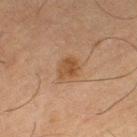biopsy_status: not biopsied; imaged during a skin examination
image:
  source: total-body photography crop
  field_of_view_mm: 15
patient:
  sex: male
  age_approx: 65
site: leg